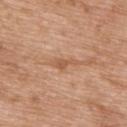follow-up = no biopsy performed (imaged during a skin exam); site = the upper back; acquisition = ~15 mm tile from a whole-body skin photo; patient = male, approximately 50 years of age; lesion diameter = ~3.5 mm (longest diameter).